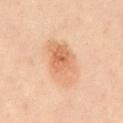Clinical impression:
This lesion was catalogued during total-body skin photography and was not selected for biopsy.
Background:
The total-body-photography lesion software estimated a lesion area of about 15 mm², a shape eccentricity near 0.85, and a shape-asymmetry score of about 0.2 (0 = symmetric). And it measured a mean CIELAB color near L≈55 a*≈19 b*≈31 and a normalized lesion–skin contrast near 6.5. And it measured a border-irregularity rating of about 2.5/10 and a peripheral color-asymmetry measure near 2. It also reported a classifier nevus-likeness of about 85/100 and a detector confidence of about 100 out of 100 that the crop contains a lesion. A male patient aged 48–52. This image is a 15 mm lesion crop taken from a total-body photograph. The lesion's longest dimension is about 6 mm. The tile uses cross-polarized illumination. From the back.